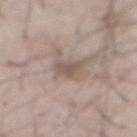  biopsy_status: not biopsied; imaged during a skin examination
  image:
    source: total-body photography crop
    field_of_view_mm: 15
  site: abdomen
  lighting: white-light
  patient:
    sex: male
    age_approx: 55
  lesion_size:
    long_diameter_mm_approx: 2.5